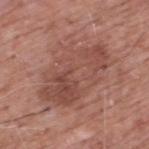notes=no biopsy performed (imaged during a skin exam)
acquisition=~15 mm crop, total-body skin-cancer survey
patient=male, aged approximately 60
location=the upper back
tile lighting=white-light illumination